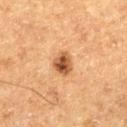Clinical impression:
Imaged during a routine full-body skin examination; the lesion was not biopsied and no histopathology is available.
Clinical summary:
The tile uses cross-polarized illumination. Longest diameter approximately 3 mm. The lesion-visualizer software estimated internal color variation of about 5.5 on a 0–10 scale and a peripheral color-asymmetry measure near 2. It also reported an automated nevus-likeness rating near 95 out of 100. A 15 mm close-up extracted from a 3D total-body photography capture. From the left thigh. A male subject, aged around 75.